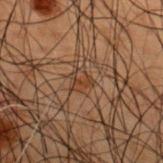Imaged during a routine full-body skin examination; the lesion was not biopsied and no histopathology is available.
Captured under cross-polarized illumination.
A male patient aged 48 to 52.
The recorded lesion diameter is about 2.5 mm.
From the upper back.
A region of skin cropped from a whole-body photographic capture, roughly 15 mm wide.